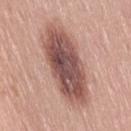| feature | finding |
|---|---|
| location | the lower back |
| size | about 10 mm |
| automated lesion analysis | a lesion color around L≈52 a*≈22 b*≈23 in CIELAB, about 16 CIELAB-L* units darker than the surrounding skin, and a normalized lesion–skin contrast near 10.5; a border-irregularity rating of about 2.5/10, a color-variation rating of about 7.5/10, and radial color variation of about 2.5; an automated nevus-likeness rating near 45 out of 100 |
| imaging modality | total-body-photography crop, ~15 mm field of view |
| patient | female, in their 40s |